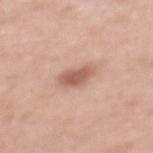This lesion was catalogued during total-body skin photography and was not selected for biopsy.
A region of skin cropped from a whole-body photographic capture, roughly 15 mm wide.
Automated tile analysis of the lesion measured an area of roughly 6.5 mm², an eccentricity of roughly 0.8, and a symmetry-axis asymmetry near 0.2. And it measured a lesion color around L≈59 a*≈22 b*≈27 in CIELAB. The software also gave a border-irregularity index near 2/10 and radial color variation of about 1. The analysis additionally found a nevus-likeness score of about 80/100 and a lesion-detection confidence of about 100/100.
The subject is a female in their mid-30s.
The lesion is located on the upper back.